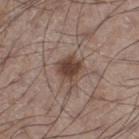Notes:
• image: 15 mm crop, total-body photography
• body site: the leg
• lighting: white-light
• lesion size: ≈4 mm
• subject: male, aged 58 to 62
• image-analysis metrics: an area of roughly 8 mm² and an outline eccentricity of about 0.6 (0 = round, 1 = elongated); an average lesion color of about L≈43 a*≈17 b*≈24 (CIELAB), a lesion–skin lightness drop of about 11, and a normalized border contrast of about 9; border irregularity of about 3.5 on a 0–10 scale, internal color variation of about 3.5 on a 0–10 scale, and peripheral color asymmetry of about 1; an automated nevus-likeness rating near 90 out of 100 and a lesion-detection confidence of about 100/100